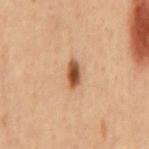Notes:
* notes · total-body-photography surveillance lesion; no biopsy
* image source · total-body-photography crop, ~15 mm field of view
* patient · male, aged approximately 60
* lesion size · ≈3 mm
* illumination · cross-polarized illumination
* body site · the mid back